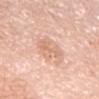Clinical impression:
Captured during whole-body skin photography for melanoma surveillance; the lesion was not biopsied.
Background:
This image is a 15 mm lesion crop taken from a total-body photograph. The lesion is on the abdomen. A male patient aged 78 to 82.